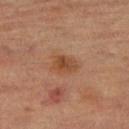The tile uses cross-polarized illumination. A male patient about 85 years old or older. Automated tile analysis of the lesion measured an area of roughly 6.5 mm², an outline eccentricity of about 0.6 (0 = round, 1 = elongated), and two-axis asymmetry of about 0.2. And it measured a mean CIELAB color near L≈40 a*≈19 b*≈29, about 7 CIELAB-L* units darker than the surrounding skin, and a lesion-to-skin contrast of about 7 (normalized; higher = more distinct). A 15 mm crop from a total-body photograph taken for skin-cancer surveillance. Located on the leg. The lesion's longest dimension is about 3 mm.